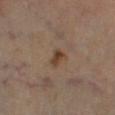| feature | finding |
|---|---|
| biopsy status | total-body-photography surveillance lesion; no biopsy |
| subject | male, in their 70s |
| lighting | cross-polarized illumination |
| body site | the leg |
| TBP lesion metrics | border irregularity of about 2.5 on a 0–10 scale and peripheral color asymmetry of about 1 |
| lesion diameter | ~2.5 mm (longest diameter) |
| image | 15 mm crop, total-body photography |